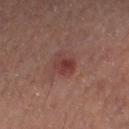The lesion was tiled from a total-body skin photograph and was not biopsied.
Cropped from a whole-body photographic skin survey; the tile spans about 15 mm.
The lesion is located on the right thigh.
Captured under cross-polarized illumination.
The lesion's longest dimension is about 3 mm.
Automated tile analysis of the lesion measured a mean CIELAB color near L≈33 a*≈22 b*≈20, about 7 CIELAB-L* units darker than the surrounding skin, and a normalized lesion–skin contrast near 7. The software also gave a border-irregularity index near 2.5/10, a color-variation rating of about 4.5/10, and peripheral color asymmetry of about 1.5. The software also gave a nevus-likeness score of about 85/100.
A male patient, approximately 50 years of age.